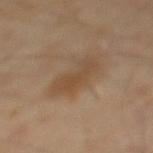imaging modality = 15 mm crop, total-body photography; patient = male, aged approximately 40; site = the mid back; size = ~4.5 mm (longest diameter).